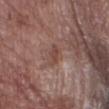This lesion was catalogued during total-body skin photography and was not selected for biopsy.
The total-body-photography lesion software estimated a color-variation rating of about 0/10 and peripheral color asymmetry of about 0. And it measured lesion-presence confidence of about 100/100.
The recorded lesion diameter is about 2.5 mm.
A male subject aged approximately 70.
A 15 mm close-up tile from a total-body photography series done for melanoma screening.
The lesion is located on the abdomen.
The tile uses white-light illumination.